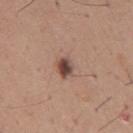Findings:
– notes: total-body-photography surveillance lesion; no biopsy
– tile lighting: white-light
– acquisition: ~15 mm tile from a whole-body skin photo
– patient: male, about 65 years old
– location: the back